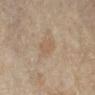Notes:
• follow-up: imaged on a skin check; not biopsied
• anatomic site: the right lower leg
• image source: 15 mm crop, total-body photography
• patient: female, aged approximately 80